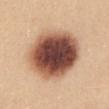Q: Was a biopsy performed?
A: catalogued during a skin exam; not biopsied
Q: What is the imaging modality?
A: ~15 mm crop, total-body skin-cancer survey
Q: Where on the body is the lesion?
A: the mid back
Q: What are the patient's age and sex?
A: female, aged 23–27